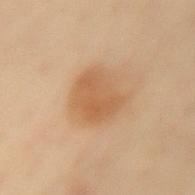This lesion was catalogued during total-body skin photography and was not selected for biopsy. Approximately 4 mm at its widest. A female subject, aged 58 to 62. Captured under cross-polarized illumination. The lesion is on the mid back. A 15 mm close-up extracted from a 3D total-body photography capture. Automated tile analysis of the lesion measured an average lesion color of about L≈51 a*≈18 b*≈33 (CIELAB). The software also gave internal color variation of about 3 on a 0–10 scale and a peripheral color-asymmetry measure near 1.